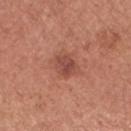Q: Was this lesion biopsied?
A: total-body-photography surveillance lesion; no biopsy
Q: Lesion size?
A: ~2.5 mm (longest diameter)
Q: How was this image acquired?
A: ~15 mm crop, total-body skin-cancer survey
Q: Who is the patient?
A: female, in their mid-60s
Q: What did automated image analysis measure?
A: an area of roughly 5 mm², an outline eccentricity of about 0.55 (0 = round, 1 = elongated), and a symmetry-axis asymmetry near 0.2; a border-irregularity index near 2/10, a color-variation rating of about 3/10, and peripheral color asymmetry of about 1
Q: Illumination type?
A: white-light
Q: Lesion location?
A: the right forearm